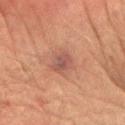site: the right forearm | image-analysis metrics: a footprint of about 5.5 mm² | tile lighting: cross-polarized | patient: male, aged 68–72 | lesion size: ≈3 mm | imaging modality: 15 mm crop, total-body photography.